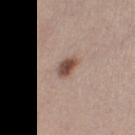notes: imaged on a skin check; not biopsied | automated lesion analysis: a mean CIELAB color near L≈49 a*≈19 b*≈25, roughly 15 lightness units darker than nearby skin, and a lesion-to-skin contrast of about 10.5 (normalized; higher = more distinct) | lesion size: ≈3 mm | acquisition: total-body-photography crop, ~15 mm field of view | body site: the right thigh | patient: female, about 40 years old.